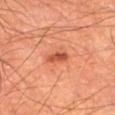Notes:
* biopsy status · total-body-photography surveillance lesion; no biopsy
* subject · male, aged 63–67
* imaging modality · 15 mm crop, total-body photography
* anatomic site · the left thigh
* tile lighting · cross-polarized illumination
* size · about 2.5 mm
* automated lesion analysis · a footprint of about 3 mm² and an outline eccentricity of about 0.85 (0 = round, 1 = elongated); an average lesion color of about L≈46 a*≈31 b*≈34 (CIELAB) and about 12 CIELAB-L* units darker than the surrounding skin; a classifier nevus-likeness of about 95/100 and a lesion-detection confidence of about 100/100